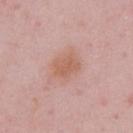A male subject aged around 50.
A lesion tile, about 15 mm wide, cut from a 3D total-body photograph.
An algorithmic analysis of the crop reported a footprint of about 7 mm², an outline eccentricity of about 0.65 (0 = round, 1 = elongated), and a shape-asymmetry score of about 0.15 (0 = symmetric).
About 3.5 mm across.
From the chest.